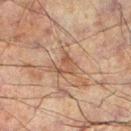lesion diameter — ≈2.5 mm
subject — male, in their 60s
lighting — cross-polarized
anatomic site — the left lower leg
image — total-body-photography crop, ~15 mm field of view
image-analysis metrics — a shape eccentricity near 0.55 and a shape-asymmetry score of about 0.45 (0 = symmetric); a mean CIELAB color near L≈40 a*≈16 b*≈25, roughly 6 lightness units darker than nearby skin, and a normalized border contrast of about 5.5; an automated nevus-likeness rating near 0 out of 100